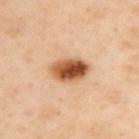Assessment:
No biopsy was performed on this lesion — it was imaged during a full skin examination and was not determined to be concerning.
Image and clinical context:
Located on the upper back. Cropped from a whole-body photographic skin survey; the tile spans about 15 mm. A male subject about 55 years old. The tile uses cross-polarized illumination. An algorithmic analysis of the crop reported an area of roughly 11 mm², an outline eccentricity of about 0.8 (0 = round, 1 = elongated), and two-axis asymmetry of about 0.15. And it measured an average lesion color of about L≈56 a*≈24 b*≈37 (CIELAB) and a normalized lesion–skin contrast near 12. The software also gave a border-irregularity rating of about 1.5/10, a color-variation rating of about 9.5/10, and peripheral color asymmetry of about 4. The software also gave a lesion-detection confidence of about 100/100. Longest diameter approximately 5 mm.